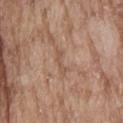Acquisition and patient details: Cropped from a whole-body photographic skin survey; the tile spans about 15 mm. On the head or neck. Measured at roughly 3 mm in maximum diameter. An algorithmic analysis of the crop reported a lesion area of about 3 mm², an eccentricity of roughly 0.95, and two-axis asymmetry of about 0.5. And it measured border irregularity of about 6 on a 0–10 scale, a color-variation rating of about 0/10, and a peripheral color-asymmetry measure near 0. Imaged with white-light lighting. A male patient about 65 years old.